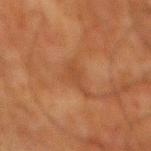Recorded during total-body skin imaging; not selected for excision or biopsy.
A male patient in their 80s.
From the mid back.
A 15 mm crop from a total-body photograph taken for skin-cancer surveillance.
Longest diameter approximately 3 mm.
Captured under cross-polarized illumination.